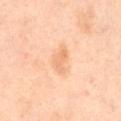Impression:
The lesion was tiled from a total-body skin photograph and was not biopsied.
Acquisition and patient details:
A male subject, aged around 45. About 3 mm across. On the left upper arm. This is a cross-polarized tile. A 15 mm close-up extracted from a 3D total-body photography capture.